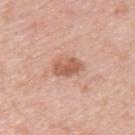Q: Was this lesion biopsied?
A: catalogued during a skin exam; not biopsied
Q: What did automated image analysis measure?
A: an area of roughly 7 mm², an outline eccentricity of about 0.8 (0 = round, 1 = elongated), and two-axis asymmetry of about 0.2; border irregularity of about 2.5 on a 0–10 scale and radial color variation of about 1; a detector confidence of about 100 out of 100 that the crop contains a lesion
Q: What is the imaging modality?
A: ~15 mm tile from a whole-body skin photo
Q: What lighting was used for the tile?
A: white-light
Q: Where on the body is the lesion?
A: the upper back
Q: Patient demographics?
A: male, aged 48 to 52